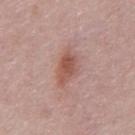Captured during whole-body skin photography for melanoma surveillance; the lesion was not biopsied. A male patient, in their mid- to late 50s. Captured under white-light illumination. Longest diameter approximately 4.5 mm. Automated tile analysis of the lesion measured a mean CIELAB color near L≈54 a*≈23 b*≈26, about 10 CIELAB-L* units darker than the surrounding skin, and a lesion-to-skin contrast of about 7.5 (normalized; higher = more distinct). The software also gave a border-irregularity index near 2.5/10, a within-lesion color-variation index near 4/10, and a peripheral color-asymmetry measure near 1. It also reported a nevus-likeness score of about 65/100 and a detector confidence of about 100 out of 100 that the crop contains a lesion. Located on the abdomen. A region of skin cropped from a whole-body photographic capture, roughly 15 mm wide.